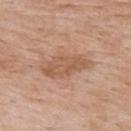The lesion was photographed on a routine skin check and not biopsied; there is no pathology result.
A roughly 15 mm field-of-view crop from a total-body skin photograph.
A female subject, in their mid-60s.
The lesion is on the upper back.
Measured at roughly 6 mm in maximum diameter.
This is a white-light tile.